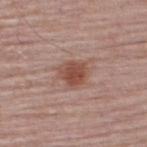Q: Is there a histopathology result?
A: imaged on a skin check; not biopsied
Q: Illumination type?
A: white-light
Q: What is the lesion's diameter?
A: about 3 mm
Q: What kind of image is this?
A: ~15 mm crop, total-body skin-cancer survey
Q: Lesion location?
A: the upper back
Q: What are the patient's age and sex?
A: male, aged approximately 65